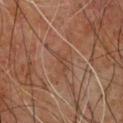The lesion was photographed on a routine skin check and not biopsied; there is no pathology result.
From the upper back.
The total-body-photography lesion software estimated roughly 5 lightness units darker than nearby skin and a lesion-to-skin contrast of about 4.5 (normalized; higher = more distinct). And it measured a border-irregularity index near 6/10, internal color variation of about 0 on a 0–10 scale, and peripheral color asymmetry of about 0. The analysis additionally found a nevus-likeness score of about 0/100 and lesion-presence confidence of about 70/100.
The recorded lesion diameter is about 2.5 mm.
The patient is a male aged approximately 60.
A roughly 15 mm field-of-view crop from a total-body skin photograph.
Imaged with cross-polarized lighting.